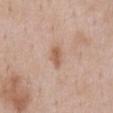Clinical impression: Recorded during total-body skin imaging; not selected for excision or biopsy. Image and clinical context: The subject is a male roughly 60 years of age. The lesion is on the abdomen. This is a white-light tile. Longest diameter approximately 3 mm. A lesion tile, about 15 mm wide, cut from a 3D total-body photograph.